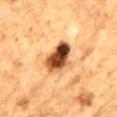follow-up = catalogued during a skin exam; not biopsied | lesion diameter = ~5 mm (longest diameter) | anatomic site = the mid back | subject = male, approximately 85 years of age or older | lighting = cross-polarized illumination | imaging modality = total-body-photography crop, ~15 mm field of view.